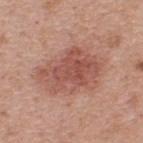Imaged during a routine full-body skin examination; the lesion was not biopsied and no histopathology is available.
A male subject, roughly 40 years of age.
On the upper back.
A roughly 15 mm field-of-view crop from a total-body skin photograph.